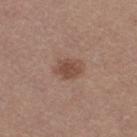Findings:
• workup — total-body-photography surveillance lesion; no biopsy
• image source — total-body-photography crop, ~15 mm field of view
• size — ~3 mm (longest diameter)
• site — the right thigh
• illumination — white-light illumination
• patient — female, in their mid- to late 30s
• TBP lesion metrics — an eccentricity of roughly 0.65 and two-axis asymmetry of about 0.25; a mean CIELAB color near L≈47 a*≈19 b*≈26, about 10 CIELAB-L* units darker than the surrounding skin, and a normalized lesion–skin contrast near 7.5; a border-irregularity index near 2/10, a within-lesion color-variation index near 2/10, and a peripheral color-asymmetry measure near 0.5